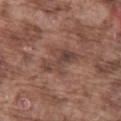follow-up: imaged on a skin check; not biopsied